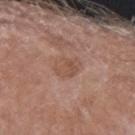Context: The recorded lesion diameter is about 3.5 mm. The lesion is on the arm. A region of skin cropped from a whole-body photographic capture, roughly 15 mm wide. Imaged with white-light lighting. A male patient in their mid- to late 80s or older.